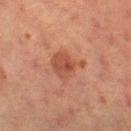The lesion was tiled from a total-body skin photograph and was not biopsied. On the left thigh. A female patient roughly 55 years of age. The recorded lesion diameter is about 4 mm. A 15 mm close-up tile from a total-body photography series done for melanoma screening. Imaged with cross-polarized lighting. The lesion-visualizer software estimated a lesion area of about 9 mm² and two-axis asymmetry of about 0.35. The analysis additionally found a border-irregularity index near 4/10, a color-variation rating of about 3/10, and peripheral color asymmetry of about 1. And it measured a classifier nevus-likeness of about 25/100 and lesion-presence confidence of about 100/100.